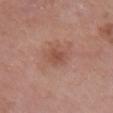{"biopsy_status": "not biopsied; imaged during a skin examination", "lesion_size": {"long_diameter_mm_approx": 3.5}, "site": "right lower leg", "patient": {"sex": "male", "age_approx": 65}, "automated_metrics": {"vs_skin_darker_L": 8.0, "vs_skin_contrast_norm": 6.0}, "image": {"source": "total-body photography crop", "field_of_view_mm": 15}}